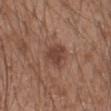Findings:
- follow-up · imaged on a skin check; not biopsied
- automated metrics · a footprint of about 7.5 mm², a shape eccentricity near 0.25, and a shape-asymmetry score of about 0.25 (0 = symmetric); a lesion–skin lightness drop of about 9 and a lesion-to-skin contrast of about 7.5 (normalized; higher = more distinct); a nevus-likeness score of about 60/100 and a lesion-detection confidence of about 100/100
- image · 15 mm crop, total-body photography
- illumination · white-light illumination
- lesion size · ~3 mm (longest diameter)
- patient · male, aged approximately 20
- anatomic site · the arm